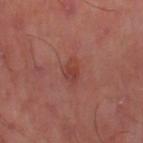The lesion was photographed on a routine skin check and not biopsied; there is no pathology result. Imaged with cross-polarized lighting. Measured at roughly 3 mm in maximum diameter. The total-body-photography lesion software estimated internal color variation of about 4 on a 0–10 scale and radial color variation of about 1.5. A region of skin cropped from a whole-body photographic capture, roughly 15 mm wide. On the left thigh.